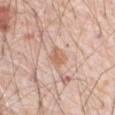notes: total-body-photography surveillance lesion; no biopsy | patient: male, aged 78 to 82 | location: the abdomen | image source: total-body-photography crop, ~15 mm field of view | lesion size: about 2.5 mm | tile lighting: white-light.